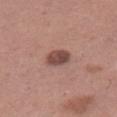{"image": {"source": "total-body photography crop", "field_of_view_mm": 15}, "site": "right thigh", "lesion_size": {"long_diameter_mm_approx": 3.0}, "automated_metrics": {"vs_skin_darker_L": 13.0, "vs_skin_contrast_norm": 9.5, "nevus_likeness_0_100": 65, "lesion_detection_confidence_0_100": 100}, "patient": {"sex": "female", "age_approx": 40}}